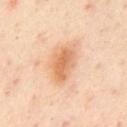No biopsy was performed on this lesion — it was imaged during a full skin examination and was not determined to be concerning. Longest diameter approximately 5 mm. A male subject, about 55 years old. Imaged with cross-polarized lighting. A 15 mm close-up extracted from a 3D total-body photography capture. Located on the chest.